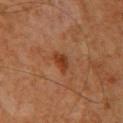Clinical impression:
No biopsy was performed on this lesion — it was imaged during a full skin examination and was not determined to be concerning.
Background:
The total-body-photography lesion software estimated a shape eccentricity near 0.75 and a shape-asymmetry score of about 0.25 (0 = symmetric). The analysis additionally found an average lesion color of about L≈35 a*≈24 b*≈32 (CIELAB) and a lesion–skin lightness drop of about 8. From the upper back. A male subject, aged approximately 60. This is a cross-polarized tile. A 15 mm close-up tile from a total-body photography series done for melanoma screening. The recorded lesion diameter is about 3 mm.